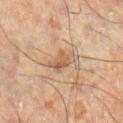workup: no biopsy performed (imaged during a skin exam) | lesion diameter: about 4 mm | image source: total-body-photography crop, ~15 mm field of view | patient: male, roughly 60 years of age | body site: the left leg | lighting: cross-polarized | image-analysis metrics: a lesion–skin lightness drop of about 7 and a normalized border contrast of about 6; a border-irregularity rating of about 3.5/10 and a peripheral color-asymmetry measure near 1; an automated nevus-likeness rating near 10 out of 100 and a detector confidence of about 95 out of 100 that the crop contains a lesion.